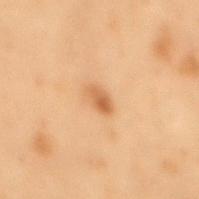Part of a total-body skin-imaging series; this lesion was reviewed on a skin check and was not flagged for biopsy.
A male patient, aged 53 to 57.
The recorded lesion diameter is about 2.5 mm.
Captured under cross-polarized illumination.
This image is a 15 mm lesion crop taken from a total-body photograph.
Located on the back.